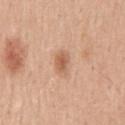On the mid back. This image is a 15 mm lesion crop taken from a total-body photograph. A male subject, approximately 50 years of age.